The subject is a male aged around 45; longest diameter approximately 6.5 mm; on the back; a 15 mm close-up extracted from a 3D total-body photography capture — 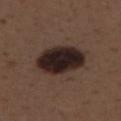pathology = an atypical melanocytic neoplasm — an indeterminate (borderline) lesion.